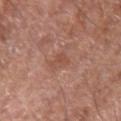Impression:
Captured during whole-body skin photography for melanoma surveillance; the lesion was not biopsied.
Clinical summary:
A male subject, aged around 80. About 3 mm across. This is a white-light tile. Automated tile analysis of the lesion measured a nevus-likeness score of about 0/100 and a lesion-detection confidence of about 100/100. Cropped from a total-body skin-imaging series; the visible field is about 15 mm. On the arm.